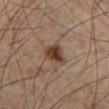A region of skin cropped from a whole-body photographic capture, roughly 15 mm wide.
The lesion's longest dimension is about 3 mm.
A male patient aged 63–67.
The lesion is on the left lower leg.
The tile uses cross-polarized illumination.
The lesion-visualizer software estimated an average lesion color of about L≈38 a*≈17 b*≈27 (CIELAB).